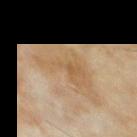Clinical impression: The lesion was photographed on a routine skin check and not biopsied; there is no pathology result. Background: A male patient, about 60 years old. Longest diameter approximately 7.5 mm. On the back. Imaged with cross-polarized lighting. A roughly 15 mm field-of-view crop from a total-body skin photograph. Automated tile analysis of the lesion measured a lesion area of about 23 mm², an outline eccentricity of about 0.8 (0 = round, 1 = elongated), and a symmetry-axis asymmetry near 0.5. The software also gave a within-lesion color-variation index near 4/10 and radial color variation of about 1. And it measured a nevus-likeness score of about 0/100 and lesion-presence confidence of about 100/100.